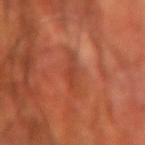site: the left forearm | imaging modality: ~15 mm tile from a whole-body skin photo | lighting: cross-polarized illumination | subject: male, in their 70s | automated lesion analysis: a footprint of about 4 mm², a shape eccentricity near 0.95, and a symmetry-axis asymmetry near 0.4; border irregularity of about 4.5 on a 0–10 scale, a within-lesion color-variation index near 2.5/10, and a peripheral color-asymmetry measure near 1; a nevus-likeness score of about 0/100 and a lesion-detection confidence of about 70/100 | size: about 4 mm.